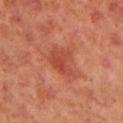Assessment:
Imaged during a routine full-body skin examination; the lesion was not biopsied and no histopathology is available.
Context:
The patient is a female about 40 years old. Approximately 3.5 mm at its widest. The lesion-visualizer software estimated an automated nevus-likeness rating near 25 out of 100. A 15 mm crop from a total-body photograph taken for skin-cancer surveillance. On the right upper arm. The tile uses cross-polarized illumination.